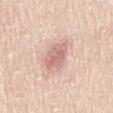{
  "lighting": "white-light",
  "image": {
    "source": "total-body photography crop",
    "field_of_view_mm": 15
  },
  "patient": {
    "sex": "female",
    "age_approx": 45
  },
  "site": "lower back"
}